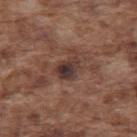Clinical impression: Captured during whole-body skin photography for melanoma surveillance; the lesion was not biopsied. Acquisition and patient details: Automated image analysis of the tile measured a border-irregularity rating of about 3/10 and peripheral color asymmetry of about 2. A male subject, in their mid- to late 70s. Longest diameter approximately 3 mm. The lesion is on the upper back. Cropped from a total-body skin-imaging series; the visible field is about 15 mm.